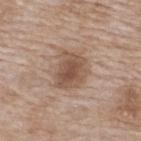Assessment:
The lesion was photographed on a routine skin check and not biopsied; there is no pathology result.
Image and clinical context:
Measured at roughly 4.5 mm in maximum diameter. The lesion is located on the upper back. A 15 mm close-up extracted from a 3D total-body photography capture. A female subject, aged 73 to 77. This is a white-light tile.